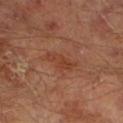notes: imaged on a skin check; not biopsied | subject: male, approximately 65 years of age | tile lighting: cross-polarized illumination | anatomic site: the left lower leg | acquisition: ~15 mm tile from a whole-body skin photo | lesion diameter: ≈4 mm.